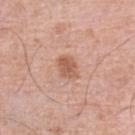Clinical impression:
No biopsy was performed on this lesion — it was imaged during a full skin examination and was not determined to be concerning.
Acquisition and patient details:
A 15 mm crop from a total-body photograph taken for skin-cancer surveillance. Automated image analysis of the tile measured a mean CIELAB color near L≈58 a*≈23 b*≈30, roughly 10 lightness units darker than nearby skin, and a normalized lesion–skin contrast near 7. The lesion is located on the left lower leg. The lesion's longest dimension is about 2.5 mm. Imaged with white-light lighting. The patient is a male aged 78 to 82.